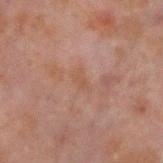biopsy_status: not biopsied; imaged during a skin examination